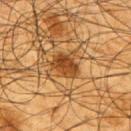No biopsy was performed on this lesion — it was imaged during a full skin examination and was not determined to be concerning.
A lesion tile, about 15 mm wide, cut from a 3D total-body photograph.
The subject is a male about 65 years old.
Located on the right upper arm.
Longest diameter approximately 3.5 mm.
Captured under cross-polarized illumination.